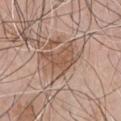<record>
<biopsy_status>not biopsied; imaged during a skin examination</biopsy_status>
<site>front of the torso</site>
<image>
  <source>total-body photography crop</source>
  <field_of_view_mm>15</field_of_view_mm>
</image>
<patient>
  <sex>male</sex>
  <age_approx>70</age_approx>
</patient>
</record>